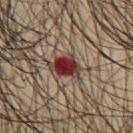The lesion was photographed on a routine skin check and not biopsied; there is no pathology result.
On the chest.
A region of skin cropped from a whole-body photographic capture, roughly 15 mm wide.
About 4 mm across.
A male patient, aged 63–67.